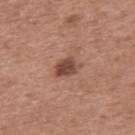The lesion was photographed on a routine skin check and not biopsied; there is no pathology result.
A male patient roughly 60 years of age.
Cropped from a whole-body photographic skin survey; the tile spans about 15 mm.
The lesion is located on the upper back.